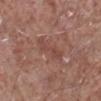This lesion was catalogued during total-body skin photography and was not selected for biopsy.